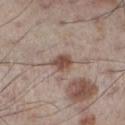Part of a total-body skin-imaging series; this lesion was reviewed on a skin check and was not flagged for biopsy.
A male subject, approximately 60 years of age.
The tile uses white-light illumination.
Cropped from a whole-body photographic skin survey; the tile spans about 15 mm.
Automated tile analysis of the lesion measured a footprint of about 4.5 mm² and a shape-asymmetry score of about 0.25 (0 = symmetric). The software also gave a mean CIELAB color near L≈49 a*≈17 b*≈24 and a normalized border contrast of about 9.
Located on the left lower leg.